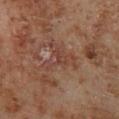| feature | finding |
|---|---|
| notes | imaged on a skin check; not biopsied |
| automated lesion analysis | a border-irregularity rating of about 7.5/10, internal color variation of about 4 on a 0–10 scale, and peripheral color asymmetry of about 1.5; a nevus-likeness score of about 0/100 and lesion-presence confidence of about 80/100 |
| site | the right lower leg |
| image source | ~15 mm tile from a whole-body skin photo |
| diameter | about 4.5 mm |
| subject | male, aged 68 to 72 |